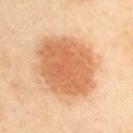Clinical impression: Part of a total-body skin-imaging series; this lesion was reviewed on a skin check and was not flagged for biopsy. Background: This is a cross-polarized tile. A 15 mm close-up tile from a total-body photography series done for melanoma screening. About 8 mm across. An algorithmic analysis of the crop reported an area of roughly 37 mm², an outline eccentricity of about 0.6 (0 = round, 1 = elongated), and a symmetry-axis asymmetry near 0.1. And it measured border irregularity of about 1.5 on a 0–10 scale, internal color variation of about 2.5 on a 0–10 scale, and peripheral color asymmetry of about 1. The lesion is located on the arm. A male patient aged around 55.